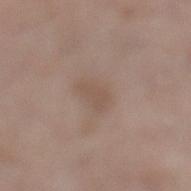This lesion was catalogued during total-body skin photography and was not selected for biopsy. A 15 mm crop from a total-body photograph taken for skin-cancer surveillance. The recorded lesion diameter is about 3 mm. From the left lower leg. A female patient approximately 60 years of age.